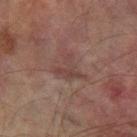Q: Was this lesion biopsied?
A: catalogued during a skin exam; not biopsied
Q: What kind of image is this?
A: 15 mm crop, total-body photography
Q: Lesion size?
A: ≈4.5 mm
Q: How was the tile lit?
A: cross-polarized illumination
Q: Automated lesion metrics?
A: an average lesion color of about L≈35 a*≈16 b*≈19 (CIELAB); a within-lesion color-variation index near 2/10 and peripheral color asymmetry of about 0.5
Q: Patient demographics?
A: male, aged 73–77
Q: Lesion location?
A: the left forearm